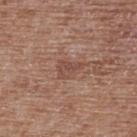This lesion was catalogued during total-body skin photography and was not selected for biopsy. This is a white-light tile. A 15 mm close-up tile from a total-body photography series done for melanoma screening. From the upper back. The lesion-visualizer software estimated a shape eccentricity near 0.8 and a shape-asymmetry score of about 0.45 (0 = symmetric). The analysis additionally found a mean CIELAB color near L≈48 a*≈21 b*≈26 and a normalized lesion–skin contrast near 6. The software also gave a classifier nevus-likeness of about 0/100. About 3 mm across. The patient is a female aged 78–82.